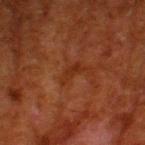| feature | finding |
|---|---|
| notes | no biopsy performed (imaged during a skin exam) |
| patient | male, roughly 80 years of age |
| anatomic site | the left thigh |
| illumination | cross-polarized illumination |
| diameter | ≈3 mm |
| imaging modality | 15 mm crop, total-body photography |
| automated lesion analysis | a lesion area of about 3 mm², a shape eccentricity near 0.9, and a shape-asymmetry score of about 0.4 (0 = symmetric); roughly 5 lightness units darker than nearby skin and a lesion-to-skin contrast of about 6 (normalized; higher = more distinct); a border-irregularity index near 5/10, a within-lesion color-variation index near 0/10, and radial color variation of about 0; a classifier nevus-likeness of about 0/100 |